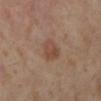Part of a total-body skin-imaging series; this lesion was reviewed on a skin check and was not flagged for biopsy. Captured under cross-polarized illumination. Measured at roughly 3 mm in maximum diameter. An algorithmic analysis of the crop reported radial color variation of about 1. The analysis additionally found lesion-presence confidence of about 100/100. The patient is a female roughly 55 years of age. A close-up tile cropped from a whole-body skin photograph, about 15 mm across. The lesion is located on the left lower leg.